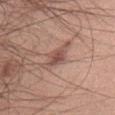The lesion was photographed on a routine skin check and not biopsied; there is no pathology result. From the chest. Imaged with white-light lighting. A male subject, roughly 30 years of age. A roughly 15 mm field-of-view crop from a total-body skin photograph. Measured at roughly 3 mm in maximum diameter.